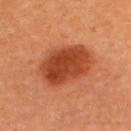Case summary:
* follow-up · imaged on a skin check; not biopsied
* site · the upper back
* image · ~15 mm tile from a whole-body skin photo
* subject · female, in their mid- to late 30s
* diameter · ~6.5 mm (longest diameter)
* illumination · cross-polarized
* image-analysis metrics · a shape-asymmetry score of about 0.15 (0 = symmetric); a border-irregularity rating of about 1.5/10 and peripheral color asymmetry of about 1.5; a detector confidence of about 100 out of 100 that the crop contains a lesion